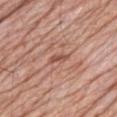Case summary:
– workup: imaged on a skin check; not biopsied
– image: ~15 mm crop, total-body skin-cancer survey
– subject: male, aged 78–82
– lesion diameter: ≈2.5 mm
– tile lighting: white-light illumination
– automated lesion analysis: a classifier nevus-likeness of about 0/100 and lesion-presence confidence of about 70/100
– site: the mid back